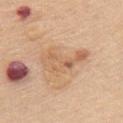  biopsy_status: not biopsied; imaged during a skin examination
  patient:
    sex: female
    age_approx: 65
  site: chest
  image:
    source: total-body photography crop
    field_of_view_mm: 15